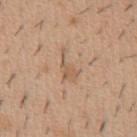Assessment: Captured during whole-body skin photography for melanoma surveillance; the lesion was not biopsied. Acquisition and patient details: Longest diameter approximately 4 mm. A male patient, approximately 40 years of age. This image is a 15 mm lesion crop taken from a total-body photograph. The lesion-visualizer software estimated a lesion area of about 4.5 mm², a shape eccentricity near 0.9, and a symmetry-axis asymmetry near 0.55. The software also gave a mean CIELAB color near L≈58 a*≈17 b*≈32, about 8 CIELAB-L* units darker than the surrounding skin, and a normalized border contrast of about 5.5. And it measured a border-irregularity index near 7/10, a color-variation rating of about 2/10, and peripheral color asymmetry of about 0.5. The software also gave an automated nevus-likeness rating near 0 out of 100 and lesion-presence confidence of about 95/100. From the abdomen. This is a white-light tile.